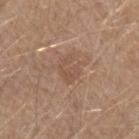biopsy status = total-body-photography surveillance lesion; no biopsy | image source = ~15 mm tile from a whole-body skin photo | patient = male, about 40 years old | location = the left forearm | size = ≈3.5 mm | illumination = white-light illumination.